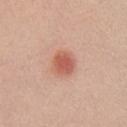Notes:
- automated metrics · roughly 11 lightness units darker than nearby skin and a normalized border contrast of about 7.5; a lesion-detection confidence of about 100/100
- imaging modality · ~15 mm crop, total-body skin-cancer survey
- patient · female, aged 18 to 22
- tile lighting · white-light illumination
- anatomic site · the front of the torso